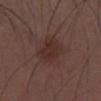<case>
  <biopsy_status>not biopsied; imaged during a skin examination</biopsy_status>
  <patient>
    <sex>male</sex>
    <age_approx>30</age_approx>
  </patient>
  <lighting>white-light</lighting>
  <image>
    <source>total-body photography crop</source>
    <field_of_view_mm>15</field_of_view_mm>
  </image>
  <automated_metrics>
    <area_mm2_approx>10.0</area_mm2_approx>
    <eccentricity>0.65</eccentricity>
    <shape_asymmetry>0.2</shape_asymmetry>
    <vs_skin_darker_L>6.0</vs_skin_darker_L>
    <vs_skin_contrast_norm>6.5</vs_skin_contrast_norm>
    <border_irregularity_0_10>2.0</border_irregularity_0_10>
    <color_variation_0_10>3.0</color_variation_0_10>
    <peripheral_color_asymmetry>1.0</peripheral_color_asymmetry>
    <nevus_likeness_0_100>5</nevus_likeness_0_100>
    <lesion_detection_confidence_0_100>100</lesion_detection_confidence_0_100>
  </automated_metrics>
  <site>chest</site>
</case>